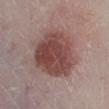Assessment:
Part of a total-body skin-imaging series; this lesion was reviewed on a skin check and was not flagged for biopsy.
Image and clinical context:
From the left lower leg. A male patient, aged approximately 80. A region of skin cropped from a whole-body photographic capture, roughly 15 mm wide.